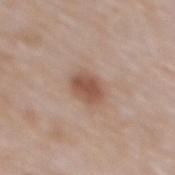The lesion was tiled from a total-body skin photograph and was not biopsied. Automated tile analysis of the lesion measured a mean CIELAB color near L≈52 a*≈20 b*≈28, roughly 12 lightness units darker than nearby skin, and a normalized border contrast of about 8.5. The analysis additionally found a nevus-likeness score of about 95/100 and lesion-presence confidence of about 100/100. From the mid back. A male patient aged around 65. Imaged with white-light lighting. The lesion's longest dimension is about 3 mm. A close-up tile cropped from a whole-body skin photograph, about 15 mm across.